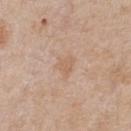* workup — no biopsy performed (imaged during a skin exam)
* image — ~15 mm crop, total-body skin-cancer survey
* site — the front of the torso
* patient — male, about 65 years old
* lighting — white-light
* diameter — ~2.5 mm (longest diameter)